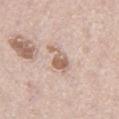Part of a total-body skin-imaging series; this lesion was reviewed on a skin check and was not flagged for biopsy. A close-up tile cropped from a whole-body skin photograph, about 15 mm across. Automated tile analysis of the lesion measured an eccentricity of roughly 0.8. The software also gave lesion-presence confidence of about 100/100. Measured at roughly 4 mm in maximum diameter. Captured under white-light illumination. The lesion is located on the left thigh. A male subject aged 63 to 67.